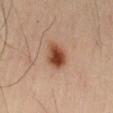Part of a total-body skin-imaging series; this lesion was reviewed on a skin check and was not flagged for biopsy. Automated tile analysis of the lesion measured a footprint of about 8 mm² and an outline eccentricity of about 0.5 (0 = round, 1 = elongated). The software also gave an automated nevus-likeness rating near 100 out of 100 and lesion-presence confidence of about 100/100. About 3.5 mm across. Imaged with cross-polarized lighting. The lesion is located on the left thigh. A male patient in their mid- to late 50s. Cropped from a whole-body photographic skin survey; the tile spans about 15 mm.